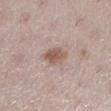The lesion was tiled from a total-body skin photograph and was not biopsied. Longest diameter approximately 3 mm. Located on the left lower leg. Captured under white-light illumination. Automated tile analysis of the lesion measured an area of roughly 5.5 mm² and a shape-asymmetry score of about 0.2 (0 = symmetric). The analysis additionally found a classifier nevus-likeness of about 65/100 and a lesion-detection confidence of about 100/100. A female subject, aged around 40. A roughly 15 mm field-of-view crop from a total-body skin photograph.